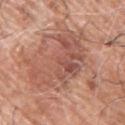The subject is a male about 60 years old.
A roughly 15 mm field-of-view crop from a total-body skin photograph.
Located on the chest.
Imaged with white-light lighting.
Approximately 7.5 mm at its widest.